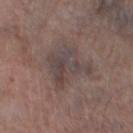Clinical impression:
Recorded during total-body skin imaging; not selected for excision or biopsy.
Clinical summary:
From the left thigh. Cropped from a whole-body photographic skin survey; the tile spans about 15 mm. The tile uses white-light illumination. The patient is a male aged 73–77. Automated tile analysis of the lesion measured a footprint of about 13 mm², a shape eccentricity near 0.75, and two-axis asymmetry of about 0.4. The analysis additionally found a border-irregularity rating of about 6/10, internal color variation of about 5.5 on a 0–10 scale, and peripheral color asymmetry of about 1.5. Measured at roughly 5.5 mm in maximum diameter.